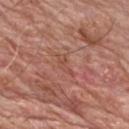  biopsy_status: not biopsied; imaged during a skin examination
  site: chest
  patient:
    sex: male
    age_approx: 60
  lesion_size:
    long_diameter_mm_approx: 3.5
  image:
    source: total-body photography crop
    field_of_view_mm: 15